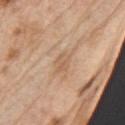Clinical impression:
Part of a total-body skin-imaging series; this lesion was reviewed on a skin check and was not flagged for biopsy.
Background:
Automated tile analysis of the lesion measured a lesion color around L≈60 a*≈18 b*≈33 in CIELAB, a lesion–skin lightness drop of about 7, and a normalized lesion–skin contrast near 5. The software also gave border irregularity of about 3 on a 0–10 scale and internal color variation of about 3 on a 0–10 scale. The software also gave a classifier nevus-likeness of about 0/100 and a lesion-detection confidence of about 95/100. The patient is a male approximately 70 years of age. On the left upper arm. A lesion tile, about 15 mm wide, cut from a 3D total-body photograph. The recorded lesion diameter is about 3 mm. The tile uses white-light illumination.